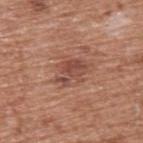Findings:
- biopsy status · total-body-photography surveillance lesion; no biopsy
- automated metrics · border irregularity of about 5 on a 0–10 scale, a within-lesion color-variation index near 3/10, and radial color variation of about 1
- patient · male, in their mid- to late 70s
- body site · the upper back
- illumination · white-light
- image · 15 mm crop, total-body photography